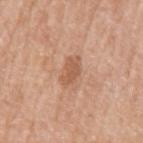This image is a 15 mm lesion crop taken from a total-body photograph.
The lesion is on the arm.
A male subject aged 68 to 72.
Longest diameter approximately 3.5 mm.
Imaged with white-light lighting.